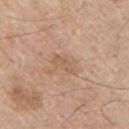<tbp_lesion>
<biopsy_status>not biopsied; imaged during a skin examination</biopsy_status>
<site>left upper arm</site>
<lesion_size>
  <long_diameter_mm_approx>3.0</long_diameter_mm_approx>
</lesion_size>
<image>
  <source>total-body photography crop</source>
  <field_of_view_mm>15</field_of_view_mm>
</image>
<patient>
  <sex>male</sex>
  <age_approx>60</age_approx>
</patient>
<lighting>white-light</lighting>
</tbp_lesion>